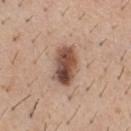{"biopsy_status": "not biopsied; imaged during a skin examination", "image": {"source": "total-body photography crop", "field_of_view_mm": 15}, "patient": {"sex": "male", "age_approx": 40}, "lighting": "white-light", "site": "front of the torso", "automated_metrics": {"eccentricity": 0.85, "shape_asymmetry": 0.15, "vs_skin_darker_L": 17.0, "border_irregularity_0_10": 2.0, "color_variation_0_10": 8.5, "nevus_likeness_0_100": 95}}